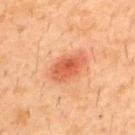biopsy status=no biopsy performed (imaged during a skin exam).